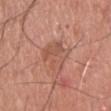biopsy_status: not biopsied; imaged during a skin examination
lighting: white-light
site: head or neck
image:
  source: total-body photography crop
  field_of_view_mm: 15
patient:
  sex: male
  age_approx: 55
automated_metrics:
  shape_asymmetry: 0.3
  border_irregularity_0_10: 3.5
  color_variation_0_10: 3.5
lesion_size:
  long_diameter_mm_approx: 4.5